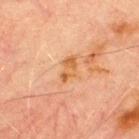Recorded during total-body skin imaging; not selected for excision or biopsy.
Captured under cross-polarized illumination.
The lesion is on the front of the torso.
A region of skin cropped from a whole-body photographic capture, roughly 15 mm wide.
The lesion's longest dimension is about 3.5 mm.
A male subject, in their 70s.